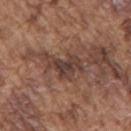{
  "biopsy_status": "not biopsied; imaged during a skin examination",
  "lighting": "white-light",
  "image": {
    "source": "total-body photography crop",
    "field_of_view_mm": 15
  },
  "patient": {
    "sex": "male",
    "age_approx": 75
  },
  "site": "mid back",
  "lesion_size": {
    "long_diameter_mm_approx": 4.0
  },
  "automated_metrics": {
    "area_mm2_approx": 7.5,
    "eccentricity": 0.8,
    "shape_asymmetry": 0.55,
    "border_irregularity_0_10": 6.5,
    "color_variation_0_10": 3.5,
    "peripheral_color_asymmetry": 1.0,
    "nevus_likeness_0_100": 0,
    "lesion_detection_confidence_0_100": 65
  }
}